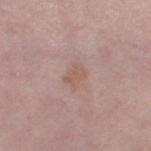biopsy status = imaged on a skin check; not biopsied | imaging modality = ~15 mm crop, total-body skin-cancer survey | subject = female, about 40 years old | tile lighting = white-light | lesion size = about 2.5 mm | body site = the left thigh.